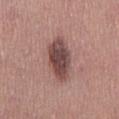Background:
A lesion tile, about 15 mm wide, cut from a 3D total-body photograph. A male subject, in their 40s. Located on the mid back.